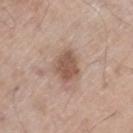This lesion was catalogued during total-body skin photography and was not selected for biopsy.